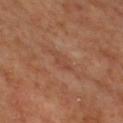Part of a total-body skin-imaging series; this lesion was reviewed on a skin check and was not flagged for biopsy. The lesion-visualizer software estimated a footprint of about 3.5 mm² and a shape-asymmetry score of about 0.35 (0 = symmetric). And it measured a classifier nevus-likeness of about 0/100 and lesion-presence confidence of about 95/100. The lesion is located on the upper back. A male patient in their mid-60s. A region of skin cropped from a whole-body photographic capture, roughly 15 mm wide.